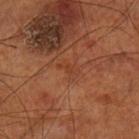notes = total-body-photography surveillance lesion; no biopsy | anatomic site = the left lower leg | acquisition = total-body-photography crop, ~15 mm field of view | TBP lesion metrics = a lesion area of about 2.5 mm², an eccentricity of roughly 0.9, and a symmetry-axis asymmetry near 0.45; an average lesion color of about L≈38 a*≈25 b*≈32 (CIELAB); a classifier nevus-likeness of about 0/100 | patient = male, aged approximately 70.